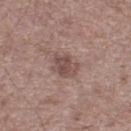Notes:
- notes: no biopsy performed (imaged during a skin exam)
- diameter: about 3.5 mm
- body site: the left thigh
- tile lighting: white-light illumination
- patient: male, aged approximately 70
- acquisition: ~15 mm tile from a whole-body skin photo
- TBP lesion metrics: a color-variation rating of about 3/10 and radial color variation of about 1; an automated nevus-likeness rating near 10 out of 100 and a detector confidence of about 100 out of 100 that the crop contains a lesion